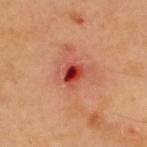Findings:
• follow-up: no biopsy performed (imaged during a skin exam)
• lesion size: ≈2.5 mm
• body site: the upper back
• patient: male, approximately 40 years of age
• tile lighting: cross-polarized illumination
• image: 15 mm crop, total-body photography
• automated lesion analysis: a mean CIELAB color near L≈45 a*≈39 b*≈36, about 15 CIELAB-L* units darker than the surrounding skin, and a normalized lesion–skin contrast near 10.5; peripheral color asymmetry of about 3; a detector confidence of about 100 out of 100 that the crop contains a lesion